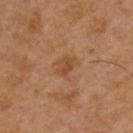biopsy status=total-body-photography surveillance lesion; no biopsy
patient=male, roughly 45 years of age
site=the back
image=~15 mm crop, total-body skin-cancer survey
lesion size=about 2.5 mm